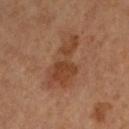Q: Is there a histopathology result?
A: no biopsy performed (imaged during a skin exam)
Q: How was the tile lit?
A: cross-polarized illumination
Q: Where on the body is the lesion?
A: the left lower leg
Q: What kind of image is this?
A: 15 mm crop, total-body photography
Q: How large is the lesion?
A: ≈7 mm
Q: Who is the patient?
A: female, in their mid-60s